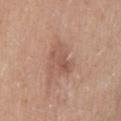biopsy status: catalogued during a skin exam; not biopsied
acquisition: ~15 mm crop, total-body skin-cancer survey
body site: the mid back
subject: female, aged around 40